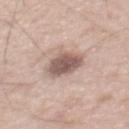biopsy status: total-body-photography surveillance lesion; no biopsy
lesion size: ~4 mm (longest diameter)
anatomic site: the back
patient: male, aged around 65
image: 15 mm crop, total-body photography
lighting: white-light illumination